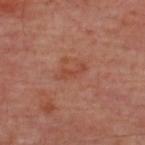No biopsy was performed on this lesion — it was imaged during a full skin examination and was not determined to be concerning.
The tile uses cross-polarized illumination.
A subject aged approximately 55.
A 15 mm crop from a total-body photograph taken for skin-cancer surveillance.
The lesion-visualizer software estimated a symmetry-axis asymmetry near 0.55. The software also gave a border-irregularity index near 6.5/10, a color-variation rating of about 0/10, and a peripheral color-asymmetry measure near 0.
Located on the upper back.